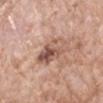A close-up tile cropped from a whole-body skin photograph, about 15 mm across.
From the left forearm.
This is a white-light tile.
A female patient about 70 years old.
Approximately 4.5 mm at its widest.